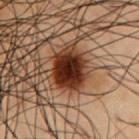Q: Is there a histopathology result?
A: no biopsy performed (imaged during a skin exam)
Q: What are the patient's age and sex?
A: male, aged around 55
Q: Lesion location?
A: the chest
Q: Lesion size?
A: ~4.5 mm (longest diameter)
Q: How was this image acquired?
A: 15 mm crop, total-body photography
Q: Automated lesion metrics?
A: a nevus-likeness score of about 100/100 and a detector confidence of about 100 out of 100 that the crop contains a lesion
Q: How was the tile lit?
A: cross-polarized illumination